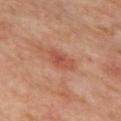Findings:
• biopsy status · total-body-photography surveillance lesion; no biopsy